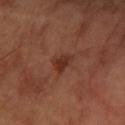follow-up: catalogued during a skin exam; not biopsied
lesion diameter: ≈2.5 mm
subject: male, in their mid-60s
imaging modality: ~15 mm tile from a whole-body skin photo
illumination: cross-polarized
site: the arm